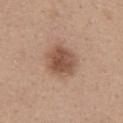<tbp_lesion>
  <patient>
    <sex>female</sex>
    <age_approx>40</age_approx>
  </patient>
  <site>upper back</site>
  <lighting>white-light</lighting>
  <image>
    <source>total-body photography crop</source>
    <field_of_view_mm>15</field_of_view_mm>
  </image>
</tbp_lesion>